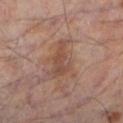This lesion was catalogued during total-body skin photography and was not selected for biopsy.
Measured at roughly 4.5 mm in maximum diameter.
A region of skin cropped from a whole-body photographic capture, roughly 15 mm wide.
The subject is a male in their mid- to late 50s.
Located on the left lower leg.
An algorithmic analysis of the crop reported an eccentricity of roughly 0.85 and a shape-asymmetry score of about 0.45 (0 = symmetric). The software also gave a lesion color around L≈44 a*≈18 b*≈25 in CIELAB, a lesion–skin lightness drop of about 8, and a lesion-to-skin contrast of about 6 (normalized; higher = more distinct).